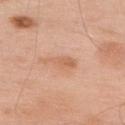Impression: The lesion was photographed on a routine skin check and not biopsied; there is no pathology result. Background: The subject is a male in their mid- to late 50s. Captured under white-light illumination. Located on the upper back. A close-up tile cropped from a whole-body skin photograph, about 15 mm across. Longest diameter approximately 4.5 mm.